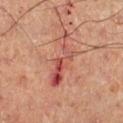This lesion was catalogued during total-body skin photography and was not selected for biopsy. The lesion is on the right lower leg. A male patient, in their 50s. A 15 mm crop from a total-body photograph taken for skin-cancer surveillance.